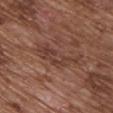Q: Was this lesion biopsied?
A: imaged on a skin check; not biopsied
Q: Who is the patient?
A: male, in their mid- to late 70s
Q: Where on the body is the lesion?
A: the chest
Q: How was this image acquired?
A: ~15 mm crop, total-body skin-cancer survey
Q: Lesion size?
A: ~6 mm (longest diameter)
Q: What lighting was used for the tile?
A: white-light illumination